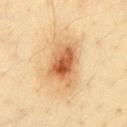Findings:
* workup — no biopsy performed (imaged during a skin exam)
* image — ~15 mm crop, total-body skin-cancer survey
* patient — male, aged around 45
* illumination — cross-polarized
* body site — the chest